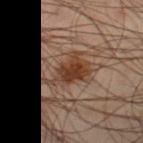notes — total-body-photography surveillance lesion; no biopsy | diameter — ≈5.5 mm | image-analysis metrics — a lesion area of about 11 mm², an outline eccentricity of about 0.75 (0 = round, 1 = elongated), and a shape-asymmetry score of about 0.45 (0 = symmetric); border irregularity of about 6 on a 0–10 scale, a within-lesion color-variation index near 5.5/10, and peripheral color asymmetry of about 1.5; an automated nevus-likeness rating near 85 out of 100 | imaging modality — ~15 mm tile from a whole-body skin photo | patient — male, aged 48 to 52 | anatomic site — the left thigh.